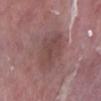Captured during whole-body skin photography for melanoma surveillance; the lesion was not biopsied. A male subject in their 40s. This image is a 15 mm lesion crop taken from a total-body photograph. On the right lower leg.